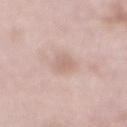The lesion was tiled from a total-body skin photograph and was not biopsied. The lesion's longest dimension is about 3.5 mm. A male patient in their mid- to late 50s. A region of skin cropped from a whole-body photographic capture, roughly 15 mm wide. The tile uses white-light illumination. On the mid back. The lesion-visualizer software estimated a lesion area of about 5 mm² and a shape eccentricity near 0.85. And it measured roughly 7 lightness units darker than nearby skin and a normalized lesion–skin contrast near 5.